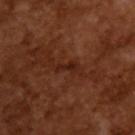No biopsy was performed on this lesion — it was imaged during a full skin examination and was not determined to be concerning.
A 15 mm close-up extracted from a 3D total-body photography capture.
The recorded lesion diameter is about 3 mm.
Automated image analysis of the tile measured a lesion area of about 2.5 mm² and a symmetry-axis asymmetry near 0.45. And it measured a mean CIELAB color near L≈22 a*≈23 b*≈26 and a normalized border contrast of about 7.5. It also reported a border-irregularity rating of about 5.5/10 and a color-variation rating of about 0/10. The analysis additionally found a classifier nevus-likeness of about 0/100.
Imaged with cross-polarized lighting.
A male subject, aged 63–67.